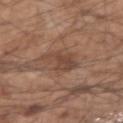The lesion was tiled from a total-body skin photograph and was not biopsied.
A male patient, in their mid- to late 50s.
The lesion-visualizer software estimated an area of roughly 8.5 mm² and a shape eccentricity near 0.75. The software also gave an automated nevus-likeness rating near 15 out of 100 and lesion-presence confidence of about 100/100.
Imaged with white-light lighting.
A 15 mm close-up extracted from a 3D total-body photography capture.
Longest diameter approximately 4 mm.
From the right forearm.